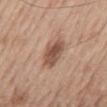- workup — no biopsy performed (imaged during a skin exam)
- body site — the mid back
- TBP lesion metrics — a lesion color around L≈52 a*≈20 b*≈28 in CIELAB, a lesion–skin lightness drop of about 13, and a normalized lesion–skin contrast near 8.5; a border-irregularity rating of about 2/10, a color-variation rating of about 4/10, and radial color variation of about 1
- patient — male, aged approximately 70
- image — total-body-photography crop, ~15 mm field of view
- lesion size — ~4 mm (longest diameter)
- illumination — white-light